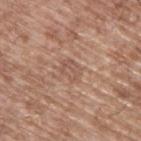notes = total-body-photography surveillance lesion; no biopsy | location = the back | subject = male, aged 63–67 | acquisition = ~15 mm crop, total-body skin-cancer survey.